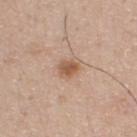Part of a total-body skin-imaging series; this lesion was reviewed on a skin check and was not flagged for biopsy. The subject is a male aged approximately 30. Approximately 2.5 mm at its widest. Automated tile analysis of the lesion measured an average lesion color of about L≈56 a*≈20 b*≈32 (CIELAB), a lesion–skin lightness drop of about 11, and a normalized lesion–skin contrast near 8. The analysis additionally found a border-irregularity index near 2/10 and internal color variation of about 3 on a 0–10 scale. It also reported an automated nevus-likeness rating near 85 out of 100 and a detector confidence of about 100 out of 100 that the crop contains a lesion. A close-up tile cropped from a whole-body skin photograph, about 15 mm across. The lesion is located on the upper back. The tile uses white-light illumination.